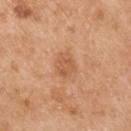<lesion>
  <biopsy_status>not biopsied; imaged during a skin examination</biopsy_status>
  <image>
    <source>total-body photography crop</source>
    <field_of_view_mm>15</field_of_view_mm>
  </image>
  <automated_metrics>
    <area_mm2_approx>5.5</area_mm2_approx>
    <eccentricity>0.65</eccentricity>
    <cielab_L>58</cielab_L>
    <cielab_a>23</cielab_a>
    <cielab_b>37</cielab_b>
    <vs_skin_darker_L>8.0</vs_skin_darker_L>
    <color_variation_0_10>2.0</color_variation_0_10>
    <peripheral_color_asymmetry>0.5</peripheral_color_asymmetry>
  </automated_metrics>
  <lighting>white-light</lighting>
  <lesion_size>
    <long_diameter_mm_approx>3.0</long_diameter_mm_approx>
  </lesion_size>
  <site>right upper arm</site>
  <patient>
    <sex>male</sex>
    <age_approx>55</age_approx>
  </patient>
</lesion>